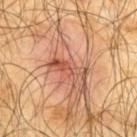workup: no biopsy performed (imaged during a skin exam) | imaging modality: ~15 mm tile from a whole-body skin photo | automated metrics: a mean CIELAB color near L≈51 a*≈25 b*≈31, a lesion–skin lightness drop of about 11, and a normalized border contrast of about 7.5; internal color variation of about 2.5 on a 0–10 scale | subject: male, aged approximately 65 | illumination: cross-polarized illumination | lesion size: about 4.5 mm | body site: the upper back.